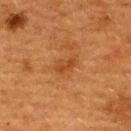Notes:
- workup · imaged on a skin check; not biopsied
- anatomic site · the upper back
- lesion size · about 2.5 mm
- illumination · cross-polarized illumination
- TBP lesion metrics · a footprint of about 3.5 mm² and two-axis asymmetry of about 0.35; about 6 CIELAB-L* units darker than the surrounding skin; a border-irregularity rating of about 3.5/10, a color-variation rating of about 1/10, and radial color variation of about 0.5; an automated nevus-likeness rating near 0 out of 100 and a detector confidence of about 100 out of 100 that the crop contains a lesion
- subject · female, roughly 40 years of age
- acquisition · 15 mm crop, total-body photography